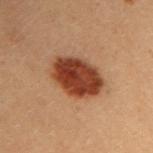Captured during whole-body skin photography for melanoma surveillance; the lesion was not biopsied.
Located on the left arm.
A 15 mm crop from a total-body photograph taken for skin-cancer surveillance.
Approximately 5.5 mm at its widest.
A female subject, aged around 30.